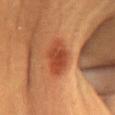Q: How was this image acquired?
A: ~15 mm tile from a whole-body skin photo
Q: Who is the patient?
A: female, approximately 30 years of age
Q: Illumination type?
A: cross-polarized
Q: Lesion location?
A: the abdomen
Q: Automated lesion metrics?
A: a lesion area of about 10 mm², an eccentricity of roughly 0.8, and a symmetry-axis asymmetry near 0.15; a lesion color around L≈44 a*≈29 b*≈36 in CIELAB and a lesion-to-skin contrast of about 8.5 (normalized; higher = more distinct); a border-irregularity index near 1.5/10; an automated nevus-likeness rating near 100 out of 100 and a detector confidence of about 100 out of 100 that the crop contains a lesion
Q: What is the lesion's diameter?
A: about 4.5 mm